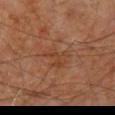- follow-up — catalogued during a skin exam; not biopsied
- subject — male, aged 58–62
- lighting — cross-polarized illumination
- automated metrics — a lesion area of about 7 mm² and an outline eccentricity of about 0.65 (0 = round, 1 = elongated); a classifier nevus-likeness of about 0/100
- site — the upper back
- lesion size — ~3.5 mm (longest diameter)
- imaging modality — ~15 mm tile from a whole-body skin photo